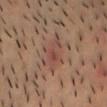<lesion>
  <biopsy_status>not biopsied; imaged during a skin examination</biopsy_status>
</lesion>